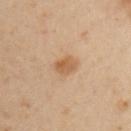No biopsy was performed on this lesion — it was imaged during a full skin examination and was not determined to be concerning.
Cropped from a whole-body photographic skin survey; the tile spans about 15 mm.
Automated image analysis of the tile measured a lesion area of about 4.5 mm² and an eccentricity of roughly 0.65. The software also gave a nevus-likeness score of about 85/100 and lesion-presence confidence of about 100/100.
A male subject in their 40s.
From the right upper arm.